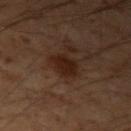No biopsy was performed on this lesion — it was imaged during a full skin examination and was not determined to be concerning. Captured under cross-polarized illumination. The lesion is on the left upper arm. A male subject aged approximately 50. A 15 mm close-up extracted from a 3D total-body photography capture. Measured at roughly 3 mm in maximum diameter.